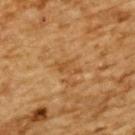  biopsy_status: not biopsied; imaged during a skin examination
  image:
    source: total-body photography crop
    field_of_view_mm: 15
  patient:
    sex: male
    age_approx: 85+
  site: upper back
  lesion_size:
    long_diameter_mm_approx: 3.0
  lighting: cross-polarized